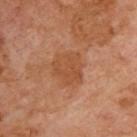Q: Is there a histopathology result?
A: no biopsy performed (imaged during a skin exam)
Q: What is the imaging modality?
A: ~15 mm crop, total-body skin-cancer survey
Q: What did automated image analysis measure?
A: a mean CIELAB color near L≈48 a*≈24 b*≈35, a lesion–skin lightness drop of about 7, and a normalized border contrast of about 6; a color-variation rating of about 2.5/10; a classifier nevus-likeness of about 15/100
Q: Where on the body is the lesion?
A: the upper back
Q: What lighting was used for the tile?
A: cross-polarized illumination
Q: Patient demographics?
A: male, approximately 70 years of age
Q: How large is the lesion?
A: ≈4 mm